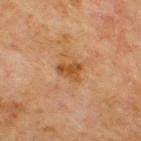No biopsy was performed on this lesion — it was imaged during a full skin examination and was not determined to be concerning.
The lesion is located on the upper back.
A male patient about 70 years old.
This image is a 15 mm lesion crop taken from a total-body photograph.